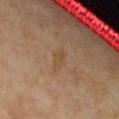Findings:
– tile lighting — cross-polarized
– site — the chest
– lesion diameter — ≈3 mm
– automated lesion analysis — a lesion-to-skin contrast of about 5.5 (normalized; higher = more distinct)
– acquisition — 15 mm crop, total-body photography
– patient — female, approximately 50 years of age